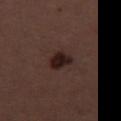Findings:
– notes · no biopsy performed (imaged during a skin exam)
– illumination · white-light illumination
– patient · female, aged around 50
– acquisition · total-body-photography crop, ~15 mm field of view
– size · about 3 mm
– anatomic site · the right thigh
– automated lesion analysis · border irregularity of about 2.5 on a 0–10 scale, internal color variation of about 3 on a 0–10 scale, and a peripheral color-asymmetry measure near 1; an automated nevus-likeness rating near 75 out of 100 and a detector confidence of about 100 out of 100 that the crop contains a lesion